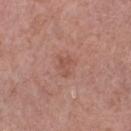Clinical summary:
The lesion's longest dimension is about 2.5 mm. The lesion is located on the arm. Automated image analysis of the tile measured a footprint of about 4 mm² and a shape-asymmetry score of about 0.45 (0 = symmetric). The analysis additionally found a within-lesion color-variation index near 1/10 and radial color variation of about 0.5. The software also gave an automated nevus-likeness rating near 0 out of 100. Imaged with white-light lighting. A female subject, in their mid- to late 60s. This image is a 15 mm lesion crop taken from a total-body photograph.